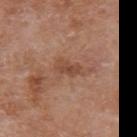| key | value |
|---|---|
| follow-up | total-body-photography surveillance lesion; no biopsy |